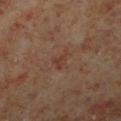notes: total-body-photography surveillance lesion; no biopsy
imaging modality: 15 mm crop, total-body photography
automated metrics: a border-irregularity rating of about 4.5/10, internal color variation of about 2 on a 0–10 scale, and a peripheral color-asymmetry measure near 0.5; a nevus-likeness score of about 0/100 and a lesion-detection confidence of about 100/100
location: the right lower leg
lesion size: about 2.5 mm
patient: male, aged 58 to 62